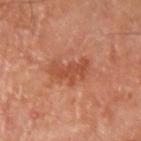{"biopsy_status": "not biopsied; imaged during a skin examination", "site": "arm", "image": {"source": "total-body photography crop", "field_of_view_mm": 15}, "patient": {"sex": "male", "age_approx": 70}, "lesion_size": {"long_diameter_mm_approx": 4.0}, "automated_metrics": {"shape_asymmetry": 0.45, "cielab_L": 48, "cielab_a": 28, "cielab_b": 34, "vs_skin_darker_L": 8.0, "vs_skin_contrast_norm": 6.5, "border_irregularity_0_10": 6.5, "color_variation_0_10": 2.0, "peripheral_color_asymmetry": 0.5, "nevus_likeness_0_100": 5}, "lighting": "cross-polarized"}